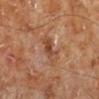Q: Was this lesion biopsied?
A: imaged on a skin check; not biopsied
Q: What is the imaging modality?
A: 15 mm crop, total-body photography
Q: Where on the body is the lesion?
A: the left lower leg
Q: What is the lesion's diameter?
A: ~4 mm (longest diameter)
Q: Who is the patient?
A: male, aged around 70
Q: What lighting was used for the tile?
A: cross-polarized illumination